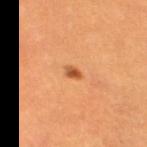<record>
<patient>
  <sex>female</sex>
  <age_approx>30</age_approx>
</patient>
<lesion_size>
  <long_diameter_mm_approx>2.0</long_diameter_mm_approx>
</lesion_size>
<site>left thigh</site>
<image>
  <source>total-body photography crop</source>
  <field_of_view_mm>15</field_of_view_mm>
</image>
</record>